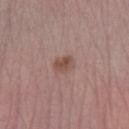Notes:
* workup: imaged on a skin check; not biopsied
* image source: ~15 mm crop, total-body skin-cancer survey
* subject: female, aged around 65
* site: the left lower leg